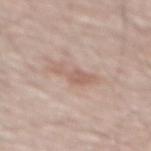This lesion was catalogued during total-body skin photography and was not selected for biopsy. A lesion tile, about 15 mm wide, cut from a 3D total-body photograph. Located on the mid back. A male subject, roughly 70 years of age. Imaged with white-light lighting.